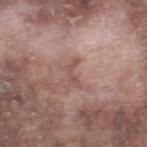* follow-up: imaged on a skin check; not biopsied
* lesion size: ~3 mm (longest diameter)
* patient: male, aged approximately 75
* image source: ~15 mm crop, total-body skin-cancer survey
* body site: the leg